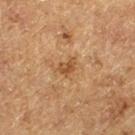<lesion>
  <lighting>cross-polarized</lighting>
  <automated_metrics>
    <cielab_L>41</cielab_L>
    <cielab_a>17</cielab_a>
    <cielab_b>32</cielab_b>
    <vs_skin_darker_L>8.0</vs_skin_darker_L>
    <vs_skin_contrast_norm>7.0</vs_skin_contrast_norm>
    <border_irregularity_0_10>4.0</border_irregularity_0_10>
  </automated_metrics>
  <image>
    <source>total-body photography crop</source>
    <field_of_view_mm>15</field_of_view_mm>
  </image>
  <site>leg</site>
  <lesion_size>
    <long_diameter_mm_approx>2.5</long_diameter_mm_approx>
  </lesion_size>
  <patient>
    <sex>male</sex>
    <age_approx>75</age_approx>
  </patient>
</lesion>